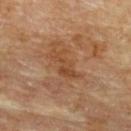lesion size=~4 mm (longest diameter); lighting=cross-polarized illumination; acquisition=total-body-photography crop, ~15 mm field of view; patient=male, aged around 85 or older; location=the upper back.